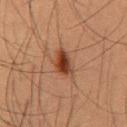Part of a total-body skin-imaging series; this lesion was reviewed on a skin check and was not flagged for biopsy.
Longest diameter approximately 4 mm.
A male patient, aged 53 to 57.
A lesion tile, about 15 mm wide, cut from a 3D total-body photograph.
The lesion is located on the mid back.